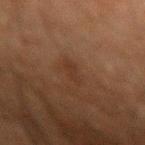biopsy_status: not biopsied; imaged during a skin examination
automated_metrics:
  eccentricity: 0.9
  shape_asymmetry: 0.3
  vs_skin_darker_L: 4.0
  vs_skin_contrast_norm: 5.0
  border_irregularity_0_10: 3.0
  color_variation_0_10: 0.0
  peripheral_color_asymmetry: 0.0
  nevus_likeness_0_100: 25
  lesion_detection_confidence_0_100: 100
patient:
  sex: male
  age_approx: 60
site: left forearm
lighting: cross-polarized
image:
  source: total-body photography crop
  field_of_view_mm: 15